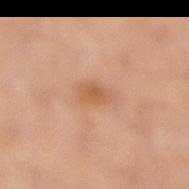{
  "biopsy_status": "not biopsied; imaged during a skin examination",
  "patient": {
    "sex": "female",
    "age_approx": 50
  },
  "site": "right lower leg",
  "lesion_size": {
    "long_diameter_mm_approx": 2.5
  },
  "automated_metrics": {
    "border_irregularity_0_10": 2.5,
    "color_variation_0_10": 1.5,
    "peripheral_color_asymmetry": 0.5
  },
  "image": {
    "source": "total-body photography crop",
    "field_of_view_mm": 15
  },
  "lighting": "cross-polarized"
}